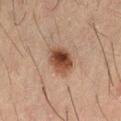A male subject in their 50s.
The tile uses cross-polarized illumination.
A close-up tile cropped from a whole-body skin photograph, about 15 mm across.
From the left thigh.